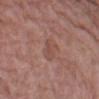Part of a total-body skin-imaging series; this lesion was reviewed on a skin check and was not flagged for biopsy. This is a white-light tile. A 15 mm close-up tile from a total-body photography series done for melanoma screening. Approximately 2.5 mm at its widest. A female subject, in their 70s. The total-body-photography lesion software estimated a footprint of about 4.5 mm². The software also gave a border-irregularity index near 3/10 and a color-variation rating of about 2/10. Located on the left thigh.